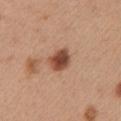This lesion was catalogued during total-body skin photography and was not selected for biopsy.
About 3 mm across.
The tile uses white-light illumination.
On the left upper arm.
A 15 mm crop from a total-body photograph taken for skin-cancer surveillance.
A female patient aged around 45.